The recorded lesion diameter is about 4 mm; the lesion is on the back; imaged with white-light lighting; a 15 mm crop from a total-body photograph taken for skin-cancer surveillance; a female subject, about 60 years old; Automated image analysis of the tile measured an area of roughly 13 mm², a shape eccentricity near 0.5, and a shape-asymmetry score of about 0.2 (0 = symmetric) — 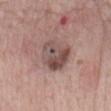Diagnosis:
On biopsy, histopathology showed an atypical melanocytic neoplasm — a lesion of indeterminate malignant potential.